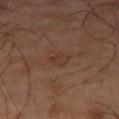Q: Is there a histopathology result?
A: total-body-photography surveillance lesion; no biopsy
Q: What kind of image is this?
A: ~15 mm crop, total-body skin-cancer survey
Q: What are the patient's age and sex?
A: male, aged 63–67
Q: What is the anatomic site?
A: the right thigh
Q: What is the lesion's diameter?
A: about 3 mm
Q: What did automated image analysis measure?
A: an average lesion color of about L≈36 a*≈19 b*≈23 (CIELAB) and a lesion-to-skin contrast of about 4.5 (normalized; higher = more distinct); a border-irregularity rating of about 3/10, internal color variation of about 4 on a 0–10 scale, and peripheral color asymmetry of about 1.5; a classifier nevus-likeness of about 0/100 and lesion-presence confidence of about 100/100